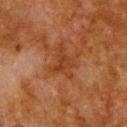| key | value |
|---|---|
| workup | total-body-photography surveillance lesion; no biopsy |
| lesion diameter | ~3.5 mm (longest diameter) |
| acquisition | ~15 mm crop, total-body skin-cancer survey |
| tile lighting | cross-polarized illumination |
| body site | the upper back |
| subject | male, aged 78 to 82 |